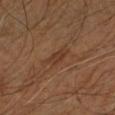Case summary:
– illumination: cross-polarized
– image source: total-body-photography crop, ~15 mm field of view
– subject: male, roughly 60 years of age
– site: the right upper arm
– lesion size: about 3 mm